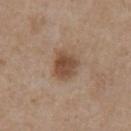{
  "biopsy_status": "not biopsied; imaged during a skin examination",
  "image": {
    "source": "total-body photography crop",
    "field_of_view_mm": 15
  },
  "automated_metrics": {
    "cielab_L": 48,
    "cielab_a": 18,
    "cielab_b": 29,
    "vs_skin_darker_L": 11.0,
    "vs_skin_contrast_norm": 8.0
  },
  "site": "chest",
  "lighting": "white-light",
  "patient": {
    "sex": "male",
    "age_approx": 65
  }
}